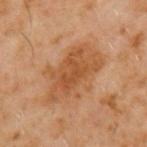The lesion was photographed on a routine skin check and not biopsied; there is no pathology result. This image is a 15 mm lesion crop taken from a total-body photograph. Approximately 8 mm at its widest. A male subject, aged 58 to 62. An algorithmic analysis of the crop reported an average lesion color of about L≈49 a*≈22 b*≈36 (CIELAB). It also reported a color-variation rating of about 4/10. And it measured an automated nevus-likeness rating near 75 out of 100 and a detector confidence of about 100 out of 100 that the crop contains a lesion. Located on the left upper arm.